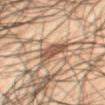{"biopsy_status": "not biopsied; imaged during a skin examination", "image": {"source": "total-body photography crop", "field_of_view_mm": 15}, "site": "mid back", "lesion_size": {"long_diameter_mm_approx": 4.0}, "patient": {"sex": "male", "age_approx": 50}, "automated_metrics": {"shape_asymmetry": 0.4, "border_irregularity_0_10": 4.5, "color_variation_0_10": 2.5}}